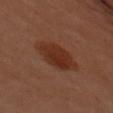Recorded during total-body skin imaging; not selected for excision or biopsy. A female patient in their 60s. A lesion tile, about 15 mm wide, cut from a 3D total-body photograph. Located on the right arm. The tile uses cross-polarized illumination. Measured at roughly 6 mm in maximum diameter. Automated image analysis of the tile measured a footprint of about 14 mm², a shape eccentricity near 0.8, and a symmetry-axis asymmetry near 0.2. It also reported an average lesion color of about L≈26 a*≈21 b*≈25 (CIELAB) and roughly 7 lightness units darker than nearby skin.